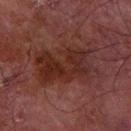biopsy status = imaged on a skin check; not biopsied
location = the right forearm
subject = male, approximately 70 years of age
tile lighting = cross-polarized illumination
image = ~15 mm crop, total-body skin-cancer survey
lesion size = about 7.5 mm